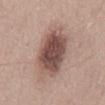Captured during whole-body skin photography for melanoma surveillance; the lesion was not biopsied. The lesion's longest dimension is about 8.5 mm. A region of skin cropped from a whole-body photographic capture, roughly 15 mm wide. A male patient, aged 23–27. The lesion is on the mid back. An algorithmic analysis of the crop reported an average lesion color of about L≈50 a*≈18 b*≈22 (CIELAB) and a lesion–skin lightness drop of about 15. The analysis additionally found a border-irregularity rating of about 2.5/10, a color-variation rating of about 6.5/10, and radial color variation of about 2. The software also gave a classifier nevus-likeness of about 90/100. Imaged with white-light lighting.